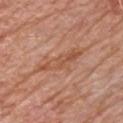Clinical summary:
The subject is a male about 80 years old. The lesion-visualizer software estimated a lesion area of about 9.5 mm², a shape eccentricity near 0.95, and two-axis asymmetry of about 0.35. The software also gave a mean CIELAB color near L≈54 a*≈24 b*≈33, a lesion–skin lightness drop of about 7, and a lesion-to-skin contrast of about 5.5 (normalized; higher = more distinct). And it measured an automated nevus-likeness rating near 0 out of 100 and lesion-presence confidence of about 70/100. About 6 mm across. On the chest. A close-up tile cropped from a whole-body skin photograph, about 15 mm across. This is a white-light tile.